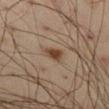Part of a total-body skin-imaging series; this lesion was reviewed on a skin check and was not flagged for biopsy.
Located on the left thigh.
A 15 mm close-up tile from a total-body photography series done for melanoma screening.
Captured under cross-polarized illumination.
A male patient, approximately 55 years of age.
Longest diameter approximately 3 mm.